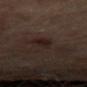Case summary:
• biopsy status — catalogued during a skin exam; not biopsied
• lighting — cross-polarized illumination
• size — ≈3 mm
• patient — male, aged 68 to 72
• anatomic site — the right thigh
• image source — ~15 mm tile from a whole-body skin photo